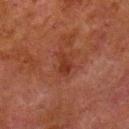| feature | finding |
|---|---|
| diameter | about 3 mm |
| automated lesion analysis | a footprint of about 3.5 mm² and a shape eccentricity near 0.85; an average lesion color of about L≈27 a*≈23 b*≈26 (CIELAB), roughly 6 lightness units darker than nearby skin, and a lesion-to-skin contrast of about 7 (normalized; higher = more distinct); border irregularity of about 3 on a 0–10 scale |
| location | the left lower leg |
| acquisition | 15 mm crop, total-body photography |
| patient | male, about 80 years old |
| lighting | cross-polarized illumination |